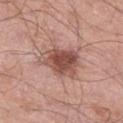Context:
About 5.5 mm across. An algorithmic analysis of the crop reported an average lesion color of about L≈51 a*≈23 b*≈25 (CIELAB), roughly 13 lightness units darker than nearby skin, and a normalized border contrast of about 9. The lesion is on the left thigh. A male subject about 70 years old. A 15 mm close-up tile from a total-body photography series done for melanoma screening. Imaged with white-light lighting.